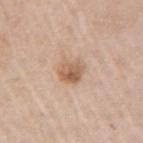Notes:
• workup · imaged on a skin check; not biopsied
• lighting · white-light
• subject · male, aged 58–62
• image source · 15 mm crop, total-body photography
• anatomic site · the right upper arm